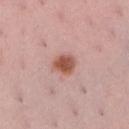The lesion was tiled from a total-body skin photograph and was not biopsied.
The lesion is on the right lower leg.
The lesion's longest dimension is about 3 mm.
The tile uses white-light illumination.
A female patient in their mid-30s.
Cropped from a whole-body photographic skin survey; the tile spans about 15 mm.